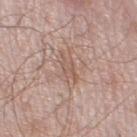Notes:
- workup · catalogued during a skin exam; not biopsied
- image source · 15 mm crop, total-body photography
- location · the left lower leg
- patient · male, roughly 55 years of age